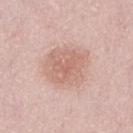biopsy status — catalogued during a skin exam; not biopsied
automated lesion analysis — a border-irregularity rating of about 3/10 and radial color variation of about 1; an automated nevus-likeness rating near 15 out of 100 and a lesion-detection confidence of about 100/100
imaging modality — ~15 mm tile from a whole-body skin photo
size — ~5 mm (longest diameter)
patient — female, aged around 50
body site — the abdomen